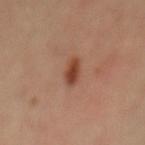Case summary:
- biopsy status · no biopsy performed (imaged during a skin exam)
- lighting · cross-polarized illumination
- automated metrics · a lesion area of about 4 mm², an outline eccentricity of about 0.85 (0 = round, 1 = elongated), and a symmetry-axis asymmetry near 0.2; a lesion color around L≈42 a*≈24 b*≈30 in CIELAB, a lesion–skin lightness drop of about 11, and a normalized lesion–skin contrast near 9.5; a detector confidence of about 100 out of 100 that the crop contains a lesion
- size · ~3 mm (longest diameter)
- image source · total-body-photography crop, ~15 mm field of view
- subject · female, about 45 years old
- body site · the lower back